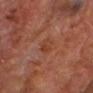Recorded during total-body skin imaging; not selected for excision or biopsy. A close-up tile cropped from a whole-body skin photograph, about 15 mm across. A male patient aged 63 to 67. The lesion is located on the head or neck.